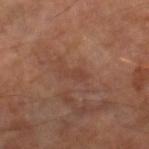workup: total-body-photography surveillance lesion; no biopsy | imaging modality: ~15 mm crop, total-body skin-cancer survey | tile lighting: cross-polarized | location: the right lower leg | patient: male, roughly 70 years of age.